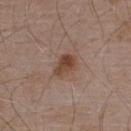{"lighting": "white-light", "site": "upper back", "automated_metrics": {"area_mm2_approx": 5.5, "eccentricity": 0.75, "shape_asymmetry": 0.25, "color_variation_0_10": 5.5, "peripheral_color_asymmetry": 2.0}, "image": {"source": "total-body photography crop", "field_of_view_mm": 15}, "patient": {"sex": "male", "age_approx": 55}, "lesion_size": {"long_diameter_mm_approx": 3.0}}